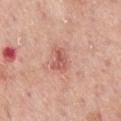<tbp_lesion>
  <biopsy_status>not biopsied; imaged during a skin examination</biopsy_status>
  <patient>
    <sex>male</sex>
    <age_approx>60</age_approx>
  </patient>
  <site>chest</site>
  <automated_metrics>
    <cielab_L>58</cielab_L>
    <cielab_a>27</cielab_a>
    <cielab_b>28</cielab_b>
    <vs_skin_darker_L>10.0</vs_skin_darker_L>
    <vs_skin_contrast_norm>6.5</vs_skin_contrast_norm>
    <border_irregularity_0_10>4.0</border_irregularity_0_10>
    <color_variation_0_10>3.5</color_variation_0_10>
    <peripheral_color_asymmetry>1.5</peripheral_color_asymmetry>
    <nevus_likeness_0_100>25</nevus_likeness_0_100>
  </automated_metrics>
  <image>
    <source>total-body photography crop</source>
    <field_of_view_mm>15</field_of_view_mm>
  </image>
  <lesion_size>
    <long_diameter_mm_approx>3.5</long_diameter_mm_approx>
  </lesion_size>
  <lighting>white-light</lighting>
</tbp_lesion>